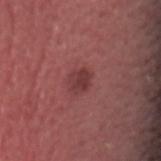  biopsy_status: not biopsied; imaged during a skin examination
  lighting: white-light
  lesion_size:
    long_diameter_mm_approx: 2.5
  site: head or neck
  patient:
    sex: male
    age_approx: 40
  image:
    source: total-body photography crop
    field_of_view_mm: 15